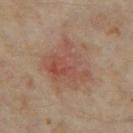patient:
  sex: female
  age_approx: 35
site: left thigh
lesion_size:
  long_diameter_mm_approx: 5.5
lighting: cross-polarized
image:
  source: total-body photography crop
  field_of_view_mm: 15
automated_metrics:
  shape_asymmetry: 0.45
  cielab_L: 47
  cielab_a: 20
  cielab_b: 26